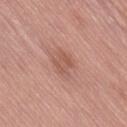Recorded during total-body skin imaging; not selected for excision or biopsy.
The lesion is located on the lower back.
An algorithmic analysis of the crop reported a mean CIELAB color near L≈55 a*≈23 b*≈28, a lesion–skin lightness drop of about 8, and a lesion-to-skin contrast of about 5.5 (normalized; higher = more distinct). And it measured a border-irregularity rating of about 3.5/10, internal color variation of about 3 on a 0–10 scale, and radial color variation of about 1. And it measured a nevus-likeness score of about 25/100 and a lesion-detection confidence of about 100/100.
The tile uses white-light illumination.
Approximately 3 mm at its widest.
Cropped from a total-body skin-imaging series; the visible field is about 15 mm.
The patient is a male in their 80s.